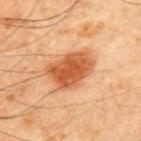workup: no biopsy performed (imaged during a skin exam) | imaging modality: ~15 mm crop, total-body skin-cancer survey | lesion size: ~5 mm (longest diameter) | body site: the chest | automated lesion analysis: a lesion–skin lightness drop of about 12 and a normalized lesion–skin contrast near 9.5; a border-irregularity rating of about 2.5/10, a color-variation rating of about 4/10, and a peripheral color-asymmetry measure near 1; a detector confidence of about 100 out of 100 that the crop contains a lesion | illumination: cross-polarized | subject: male, aged around 70.